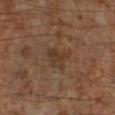No biopsy was performed on this lesion — it was imaged during a full skin examination and was not determined to be concerning.
The lesion-visualizer software estimated a border-irregularity index near 4/10, internal color variation of about 1.5 on a 0–10 scale, and radial color variation of about 0.5.
Imaged with cross-polarized lighting.
Cropped from a whole-body photographic skin survey; the tile spans about 15 mm.
Located on the right lower leg.
A male subject, aged 58–62.